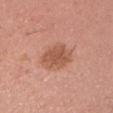The lesion was tiled from a total-body skin photograph and was not biopsied. Located on the head or neck. A 15 mm close-up tile from a total-body photography series done for melanoma screening. A female patient aged around 25.